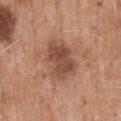patient: male, approximately 70 years of age; site: the mid back; image: 15 mm crop, total-body photography; illumination: white-light illumination.